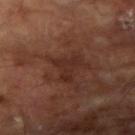Assessment:
This lesion was catalogued during total-body skin photography and was not selected for biopsy.
Image and clinical context:
On the right upper arm. This image is a 15 mm lesion crop taken from a total-body photograph. A male subject in their mid- to late 60s. Imaged with cross-polarized lighting.